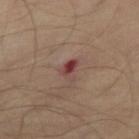biopsy status = total-body-photography surveillance lesion; no biopsy | lighting = cross-polarized | subject = male, aged approximately 70 | site = the abdomen | image source = ~15 mm tile from a whole-body skin photo | automated metrics = an area of roughly 3.5 mm², a shape eccentricity near 0.75, and a symmetry-axis asymmetry near 0.45; border irregularity of about 4 on a 0–10 scale, a color-variation rating of about 3.5/10, and a peripheral color-asymmetry measure near 1.